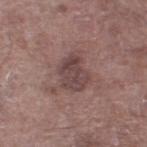workup: no biopsy performed (imaged during a skin exam) | image source: 15 mm crop, total-body photography | subject: male, approximately 70 years of age | diameter: ~4 mm (longest diameter) | site: the left thigh.